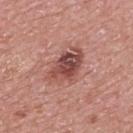Part of a total-body skin-imaging series; this lesion was reviewed on a skin check and was not flagged for biopsy. Approximately 5 mm at its widest. Captured under white-light illumination. A lesion tile, about 15 mm wide, cut from a 3D total-body photograph. From the mid back. Automated image analysis of the tile measured a footprint of about 12 mm² and a shape eccentricity near 0.8. The software also gave a lesion color around L≈48 a*≈25 b*≈24 in CIELAB, about 13 CIELAB-L* units darker than the surrounding skin, and a normalized lesion–skin contrast near 9. And it measured a border-irregularity index near 3.5/10, a color-variation rating of about 6.5/10, and a peripheral color-asymmetry measure near 2. A male patient about 70 years old.